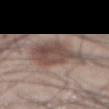No biopsy was performed on this lesion — it was imaged during a full skin examination and was not determined to be concerning. A close-up tile cropped from a whole-body skin photograph, about 15 mm across. On the abdomen. A male patient, aged 43–47. The lesion-visualizer software estimated a mean CIELAB color near L≈47 a*≈15 b*≈21, about 11 CIELAB-L* units darker than the surrounding skin, and a lesion-to-skin contrast of about 8 (normalized; higher = more distinct). The analysis additionally found a color-variation rating of about 4/10 and radial color variation of about 1.5. The software also gave a classifier nevus-likeness of about 20/100 and lesion-presence confidence of about 75/100. Measured at roughly 5 mm in maximum diameter.